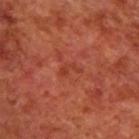Part of a total-body skin-imaging series; this lesion was reviewed on a skin check and was not flagged for biopsy.
A male subject, aged around 70.
The lesion is located on the upper back.
This image is a 15 mm lesion crop taken from a total-body photograph.